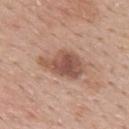Part of a total-body skin-imaging series; this lesion was reviewed on a skin check and was not flagged for biopsy.
Approximately 5.5 mm at its widest.
A roughly 15 mm field-of-view crop from a total-body skin photograph.
Captured under white-light illumination.
On the back.
A male patient, about 55 years old.